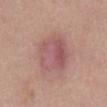Q: Was this lesion biopsied?
A: total-body-photography surveillance lesion; no biopsy
Q: Patient demographics?
A: female, about 40 years old
Q: How was this image acquired?
A: ~15 mm crop, total-body skin-cancer survey
Q: Automated lesion metrics?
A: an outline eccentricity of about 0.6 (0 = round, 1 = elongated) and two-axis asymmetry of about 0.2; an average lesion color of about L≈55 a*≈23 b*≈21 (CIELAB), a lesion–skin lightness drop of about 8, and a normalized border contrast of about 6; a border-irregularity index near 2/10, a color-variation rating of about 6/10, and peripheral color asymmetry of about 2; a classifier nevus-likeness of about 0/100 and a detector confidence of about 100 out of 100 that the crop contains a lesion
Q: How was the tile lit?
A: white-light illumination
Q: Lesion location?
A: the abdomen